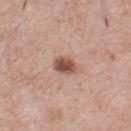Q: Was a biopsy performed?
A: no biopsy performed (imaged during a skin exam)
Q: Who is the patient?
A: male, aged approximately 40
Q: What did automated image analysis measure?
A: a lesion-detection confidence of about 100/100
Q: Lesion location?
A: the front of the torso
Q: How was this image acquired?
A: total-body-photography crop, ~15 mm field of view
Q: Illumination type?
A: white-light illumination